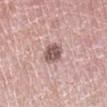Notes:
- workup · catalogued during a skin exam; not biopsied
- patient · male, aged around 50
- body site · the right lower leg
- tile lighting · white-light
- imaging modality · ~15 mm tile from a whole-body skin photo
- size · ≈3 mm